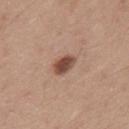Assessment: The lesion was photographed on a routine skin check and not biopsied; there is no pathology result. Acquisition and patient details: This is a white-light tile. Cropped from a total-body skin-imaging series; the visible field is about 15 mm. On the mid back. Approximately 3 mm at its widest. An algorithmic analysis of the crop reported a lesion color around L≈47 a*≈21 b*≈27 in CIELAB, about 15 CIELAB-L* units darker than the surrounding skin, and a lesion-to-skin contrast of about 10.5 (normalized; higher = more distinct). The analysis additionally found an automated nevus-likeness rating near 95 out of 100. The subject is a male aged around 35.